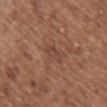Clinical summary: A lesion tile, about 15 mm wide, cut from a 3D total-body photograph. The lesion is on the upper back. Measured at roughly 3 mm in maximum diameter. A female subject approximately 75 years of age. This is a white-light tile.